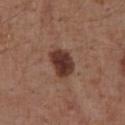| feature | finding |
|---|---|
| biopsy status | total-body-photography surveillance lesion; no biopsy |
| subject | male, in their mid-50s |
| body site | the abdomen |
| lesion diameter | ~3.5 mm (longest diameter) |
| image source | ~15 mm tile from a whole-body skin photo |
| illumination | white-light |